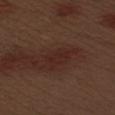Captured during whole-body skin photography for melanoma surveillance; the lesion was not biopsied. Imaged with white-light lighting. From the left upper arm. The patient is a male about 70 years old. Cropped from a total-body skin-imaging series; the visible field is about 15 mm.